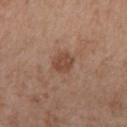Impression: Part of a total-body skin-imaging series; this lesion was reviewed on a skin check and was not flagged for biopsy. Background: Located on the mid back. A region of skin cropped from a whole-body photographic capture, roughly 15 mm wide. The lesion-visualizer software estimated a lesion area of about 4.5 mm² and a shape-asymmetry score of about 0.2 (0 = symmetric). It also reported a mean CIELAB color near L≈46 a*≈20 b*≈29, roughly 9 lightness units darker than nearby skin, and a normalized border contrast of about 7. It also reported internal color variation of about 2 on a 0–10 scale and peripheral color asymmetry of about 0.5. A male subject roughly 55 years of age. The lesion's longest dimension is about 2.5 mm. Imaged with white-light lighting.